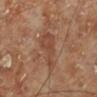biopsy status: no biopsy performed (imaged during a skin exam) | subject: male, about 70 years old | image: 15 mm crop, total-body photography | illumination: cross-polarized | location: the left lower leg.